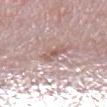An algorithmic analysis of the crop reported a mean CIELAB color near L≈58 a*≈20 b*≈23, about 9 CIELAB-L* units darker than the surrounding skin, and a normalized lesion–skin contrast near 6.5. The analysis additionally found a border-irregularity index near 3/10, a color-variation rating of about 2.5/10, and a peripheral color-asymmetry measure near 0.5.
On the left lower leg.
A female patient, about 40 years old.
A 15 mm close-up extracted from a 3D total-body photography capture.
The tile uses white-light illumination.